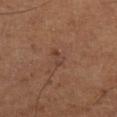<record>
<image>
  <source>total-body photography crop</source>
  <field_of_view_mm>15</field_of_view_mm>
</image>
<patient>
  <sex>male</sex>
  <age_approx>60</age_approx>
</patient>
<site>left lower leg</site>
<automated_metrics>
  <area_mm2_approx>2.5</area_mm2_approx>
  <eccentricity>0.9</eccentricity>
  <shape_asymmetry>0.35</shape_asymmetry>
  <cielab_L>39</cielab_L>
  <cielab_a>20</cielab_a>
  <cielab_b>26</cielab_b>
  <vs_skin_darker_L>6.0</vs_skin_darker_L>
  <vs_skin_contrast_norm>5.5</vs_skin_contrast_norm>
  <lesion_detection_confidence_0_100>100</lesion_detection_confidence_0_100>
</automated_metrics>
</record>